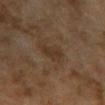follow-up = total-body-photography surveillance lesion; no biopsy
body site = the arm
subject = female, aged 58 to 62
image = ~15 mm tile from a whole-body skin photo